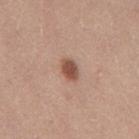The lesion was photographed on a routine skin check and not biopsied; there is no pathology result.
The lesion's longest dimension is about 2.5 mm.
Imaged with white-light lighting.
A male patient aged approximately 25.
Located on the back.
A 15 mm close-up tile from a total-body photography series done for melanoma screening.